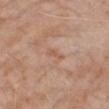Background: A region of skin cropped from a whole-body photographic capture, roughly 15 mm wide. A male subject, roughly 75 years of age. The lesion is located on the arm.